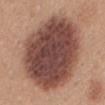The subject is a female roughly 30 years of age.
Measured at roughly 11 mm in maximum diameter.
The lesion is on the back.
The tile uses white-light illumination.
Cropped from a whole-body photographic skin survey; the tile spans about 15 mm.
An algorithmic analysis of the crop reported an area of roughly 70 mm² and a symmetry-axis asymmetry near 0.1.